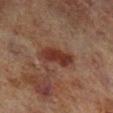Findings:
– image source — 15 mm crop, total-body photography
– site — the right lower leg
– patient — male, approximately 70 years of age
– lighting — cross-polarized illumination
– lesion size — about 4.5 mm